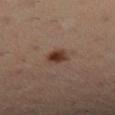Captured during whole-body skin photography for melanoma surveillance; the lesion was not biopsied. The tile uses cross-polarized illumination. A female patient about 35 years old. Longest diameter approximately 2.5 mm. A close-up tile cropped from a whole-body skin photograph, about 15 mm across. Located on the left lower leg.